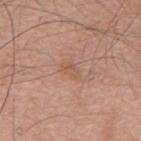Background: The recorded lesion diameter is about 3 mm. From the mid back. A male subject, aged 73–77. A roughly 15 mm field-of-view crop from a total-body skin photograph. This is a white-light tile.